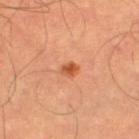Clinical impression:
Part of a total-body skin-imaging series; this lesion was reviewed on a skin check and was not flagged for biopsy.
Acquisition and patient details:
The subject is a male aged 63–67. A roughly 15 mm field-of-view crop from a total-body skin photograph. Located on the left thigh.